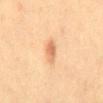Part of a total-body skin-imaging series; this lesion was reviewed on a skin check and was not flagged for biopsy. A 15 mm close-up extracted from a 3D total-body photography capture. The tile uses cross-polarized illumination. A male subject, roughly 40 years of age. The recorded lesion diameter is about 3 mm. On the mid back. The total-body-photography lesion software estimated a lesion area of about 4 mm², an outline eccentricity of about 0.85 (0 = round, 1 = elongated), and a symmetry-axis asymmetry near 0.15.